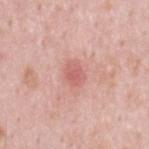Assessment:
Captured during whole-body skin photography for melanoma surveillance; the lesion was not biopsied.
Image and clinical context:
Captured under white-light illumination. The patient is a male approximately 35 years of age. The lesion is located on the back. A 15 mm crop from a total-body photograph taken for skin-cancer surveillance.